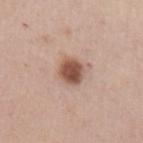biopsy status = no biopsy performed (imaged during a skin exam) | patient = male, aged 58–62 | image source = ~15 mm crop, total-body skin-cancer survey | anatomic site = the left upper arm | illumination = white-light | lesion diameter = ~3 mm (longest diameter).